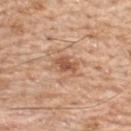Captured during whole-body skin photography for melanoma surveillance; the lesion was not biopsied. The lesion's longest dimension is about 3 mm. A male subject, roughly 60 years of age. Automated image analysis of the tile measured a lesion area of about 5.5 mm², a shape eccentricity near 0.75, and a symmetry-axis asymmetry near 0.25. And it measured roughly 11 lightness units darker than nearby skin. The software also gave border irregularity of about 3 on a 0–10 scale and radial color variation of about 1. Imaged with white-light lighting. Cropped from a whole-body photographic skin survey; the tile spans about 15 mm. Located on the upper back.